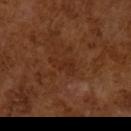<record>
  <biopsy_status>not biopsied; imaged during a skin examination</biopsy_status>
  <image>
    <source>total-body photography crop</source>
    <field_of_view_mm>15</field_of_view_mm>
  </image>
  <lighting>cross-polarized</lighting>
  <lesion_size>
    <long_diameter_mm_approx>3.5</long_diameter_mm_approx>
  </lesion_size>
  <automated_metrics>
    <area_mm2_approx>4.0</area_mm2_approx>
    <eccentricity>0.85</eccentricity>
    <shape_asymmetry>0.5</shape_asymmetry>
    <peripheral_color_asymmetry>0.0</peripheral_color_asymmetry>
    <lesion_detection_confidence_0_100>100</lesion_detection_confidence_0_100>
  </automated_metrics>
  <patient>
    <sex>male</sex>
    <age_approx>65</age_approx>
  </patient>
</record>